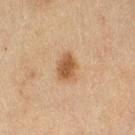No biopsy was performed on this lesion — it was imaged during a full skin examination and was not determined to be concerning. From the right thigh. A female patient, aged 53 to 57. Approximately 3.5 mm at its widest. Cropped from a whole-body photographic skin survey; the tile spans about 15 mm. Captured under cross-polarized illumination.